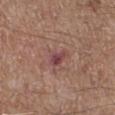• biopsy status — total-body-photography surveillance lesion; no biopsy
• site — the right lower leg
• subject — male, aged 53 to 57
• illumination — white-light
• image source — ~15 mm tile from a whole-body skin photo
• image-analysis metrics — a lesion area of about 4.5 mm², a shape eccentricity near 0.8, and a symmetry-axis asymmetry near 0.4; roughly 9 lightness units darker than nearby skin and a lesion-to-skin contrast of about 8 (normalized; higher = more distinct); peripheral color asymmetry of about 1.5; a nevus-likeness score of about 0/100 and a detector confidence of about 100 out of 100 that the crop contains a lesion
• lesion diameter — ~3 mm (longest diameter)